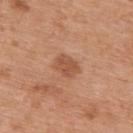{"biopsy_status": "not biopsied; imaged during a skin examination", "patient": {"sex": "male", "age_approx": 70}, "image": {"source": "total-body photography crop", "field_of_view_mm": 15}, "lesion_size": {"long_diameter_mm_approx": 3.0}, "lighting": "white-light", "site": "upper back"}